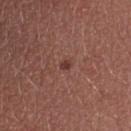This lesion was catalogued during total-body skin photography and was not selected for biopsy. The subject is a female aged 23–27. Approximately 1.5 mm at its widest. From the upper back. The tile uses white-light illumination. A roughly 15 mm field-of-view crop from a total-body skin photograph.